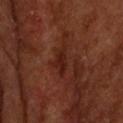The tile uses cross-polarized illumination. A male subject, aged 63 to 67. On the upper back. A close-up tile cropped from a whole-body skin photograph, about 15 mm across.